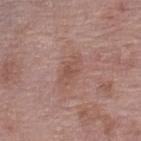<tbp_lesion>
<biopsy_status>not biopsied; imaged during a skin examination</biopsy_status>
<patient>
  <sex>female</sex>
  <age_approx>70</age_approx>
</patient>
<lighting>white-light</lighting>
<image>
  <source>total-body photography crop</source>
  <field_of_view_mm>15</field_of_view_mm>
</image>
<site>left lower leg</site>
<automated_metrics>
  <area_mm2_approx>5.5</area_mm2_approx>
  <shape_asymmetry>0.25</shape_asymmetry>
  <cielab_L>52</cielab_L>
  <cielab_a>20</cielab_a>
  <cielab_b>25</cielab_b>
  <vs_skin_darker_L>7.0</vs_skin_darker_L>
  <vs_skin_contrast_norm>5.5</vs_skin_contrast_norm>
</automated_metrics>
</tbp_lesion>